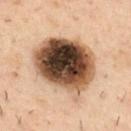Impression: Recorded during total-body skin imaging; not selected for excision or biopsy. Background: The subject is a male in their 40s. An algorithmic analysis of the crop reported a shape eccentricity near 0.6 and a symmetry-axis asymmetry near 0.15. The software also gave an average lesion color of about L≈46 a*≈17 b*≈29 (CIELAB) and about 27 CIELAB-L* units darker than the surrounding skin. The tile uses cross-polarized illumination. Located on the upper back. A lesion tile, about 15 mm wide, cut from a 3D total-body photograph. Approximately 8 mm at its widest.